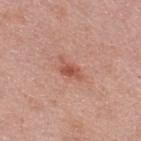Imaged during a routine full-body skin examination; the lesion was not biopsied and no histopathology is available. On the upper back. Approximately 3.5 mm at its widest. The total-body-photography lesion software estimated a footprint of about 4 mm², an outline eccentricity of about 0.85 (0 = round, 1 = elongated), and two-axis asymmetry of about 0.45. The software also gave a border-irregularity index near 5/10 and peripheral color asymmetry of about 0.5. This is a white-light tile. A male subject in their mid-40s. A 15 mm close-up tile from a total-body photography series done for melanoma screening.